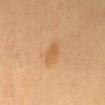– workup: total-body-photography surveillance lesion; no biopsy
– lesion diameter: ≈3 mm
– location: the mid back
– subject: female, aged 48 to 52
– acquisition: ~15 mm tile from a whole-body skin photo
– illumination: cross-polarized